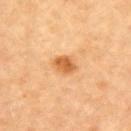No biopsy was performed on this lesion — it was imaged during a full skin examination and was not determined to be concerning. Longest diameter approximately 2.5 mm. Automated image analysis of the tile measured a footprint of about 5 mm² and an eccentricity of roughly 0.65. The analysis additionally found a mean CIELAB color near L≈61 a*≈26 b*≈44 and a normalized lesion–skin contrast near 8. The software also gave a color-variation rating of about 3/10. A male subject roughly 85 years of age or older. Imaged with cross-polarized lighting. The lesion is on the left upper arm. A 15 mm close-up extracted from a 3D total-body photography capture.